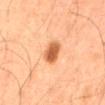Assessment:
The lesion was tiled from a total-body skin photograph and was not biopsied.
Clinical summary:
An algorithmic analysis of the crop reported border irregularity of about 2 on a 0–10 scale, a within-lesion color-variation index near 3.5/10, and radial color variation of about 1. The software also gave a classifier nevus-likeness of about 100/100 and a detector confidence of about 100 out of 100 that the crop contains a lesion. A male patient, aged 63 to 67. The recorded lesion diameter is about 3 mm. The lesion is located on the mid back. A 15 mm crop from a total-body photograph taken for skin-cancer surveillance.